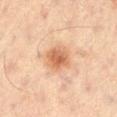{"biopsy_status": "not biopsied; imaged during a skin examination", "site": "left thigh", "patient": {"sex": "male", "age_approx": 60}, "lesion_size": {"long_diameter_mm_approx": 3.5}, "lighting": "cross-polarized", "image": {"source": "total-body photography crop", "field_of_view_mm": 15}}